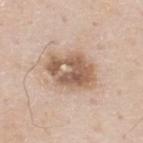No biopsy was performed on this lesion — it was imaged during a full skin examination and was not determined to be concerning. Imaged with white-light lighting. An algorithmic analysis of the crop reported an outline eccentricity of about 0.75 (0 = round, 1 = elongated) and two-axis asymmetry of about 0.2. It also reported a lesion color around L≈59 a*≈17 b*≈29 in CIELAB, a lesion–skin lightness drop of about 13, and a lesion-to-skin contrast of about 8.5 (normalized; higher = more distinct). The software also gave radial color variation of about 3.5. On the upper back. A close-up tile cropped from a whole-body skin photograph, about 15 mm across. The recorded lesion diameter is about 5.5 mm. A male patient aged 78 to 82.